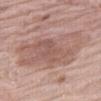follow-up = imaged on a skin check; not biopsied | subject = male, roughly 80 years of age | site = the right thigh | illumination = white-light | automated metrics = a border-irregularity index near 4/10, a within-lesion color-variation index near 4.5/10, and a peripheral color-asymmetry measure near 1.5; a classifier nevus-likeness of about 0/100 and a lesion-detection confidence of about 100/100 | acquisition = total-body-photography crop, ~15 mm field of view | diameter = ≈9.5 mm.